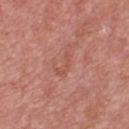site: the chest
patient: male, approximately 70 years of age
imaging modality: 15 mm crop, total-body photography